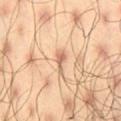Clinical impression:
The lesion was photographed on a routine skin check and not biopsied; there is no pathology result.
Image and clinical context:
Imaged with cross-polarized lighting. Measured at roughly 3 mm in maximum diameter. The total-body-photography lesion software estimated an area of roughly 2.5 mm² and a shape eccentricity near 0.9. The software also gave a border-irregularity rating of about 6.5/10, a color-variation rating of about 0.5/10, and peripheral color asymmetry of about 0. The analysis additionally found a classifier nevus-likeness of about 0/100 and lesion-presence confidence of about 100/100. A male patient, aged 43 to 47. From the right thigh. A 15 mm crop from a total-body photograph taken for skin-cancer surveillance.